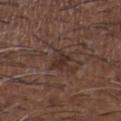notes: imaged on a skin check; not biopsied | TBP lesion metrics: a lesion color around L≈30 a*≈16 b*≈20 in CIELAB, about 8 CIELAB-L* units darker than the surrounding skin, and a normalized lesion–skin contrast near 8; border irregularity of about 4 on a 0–10 scale and a within-lesion color-variation index near 2/10 | body site: the back | subject: male, aged approximately 50 | diameter: about 3.5 mm | acquisition: 15 mm crop, total-body photography.